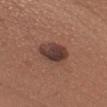Acquisition and patient details:
A 15 mm close-up tile from a total-body photography series done for melanoma screening. Automated image analysis of the tile measured an area of roughly 11 mm² and two-axis asymmetry of about 0.15. And it measured a border-irregularity rating of about 1.5/10, internal color variation of about 5.5 on a 0–10 scale, and peripheral color asymmetry of about 2. The software also gave a classifier nevus-likeness of about 60/100 and lesion-presence confidence of about 100/100. This is a white-light tile. The lesion is located on the chest. A male patient, about 35 years old.